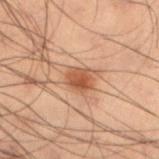Imaged during a routine full-body skin examination; the lesion was not biopsied and no histopathology is available. The lesion is located on the left lower leg. A 15 mm crop from a total-body photograph taken for skin-cancer surveillance. A male patient, about 55 years old. This is a cross-polarized tile. Measured at roughly 2.5 mm in maximum diameter.